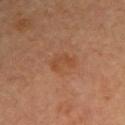The lesion was photographed on a routine skin check and not biopsied; there is no pathology result. This is a cross-polarized tile. Cropped from a total-body skin-imaging series; the visible field is about 15 mm. Located on the right upper arm. A male subject, aged 63 to 67.